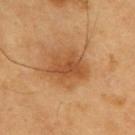Case summary:
• imaging modality — total-body-photography crop, ~15 mm field of view
• lighting — cross-polarized illumination
• anatomic site — the upper back
• subject — male, in their 60s
• diameter — ≈5 mm